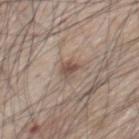Captured during whole-body skin photography for melanoma surveillance; the lesion was not biopsied. The patient is a male approximately 65 years of age. Automated image analysis of the tile measured a lesion color around L≈51 a*≈15 b*≈24 in CIELAB, roughly 10 lightness units darker than nearby skin, and a lesion-to-skin contrast of about 7.5 (normalized; higher = more distinct). The software also gave lesion-presence confidence of about 100/100. From the chest. A region of skin cropped from a whole-body photographic capture, roughly 15 mm wide.